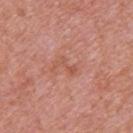No biopsy was performed on this lesion — it was imaged during a full skin examination and was not determined to be concerning. The lesion is on the back. Measured at roughly 3 mm in maximum diameter. This is a white-light tile. A 15 mm close-up tile from a total-body photography series done for melanoma screening. A female subject about 40 years old.